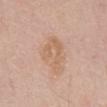Clinical impression: The lesion was tiled from a total-body skin photograph and was not biopsied. Clinical summary: The lesion is located on the abdomen. Imaged with white-light lighting. A male subject, aged 68–72. The recorded lesion diameter is about 5 mm. A 15 mm crop from a total-body photograph taken for skin-cancer surveillance.